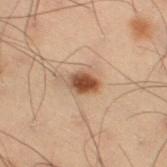Q: Is there a histopathology result?
A: no biopsy performed (imaged during a skin exam)
Q: Where on the body is the lesion?
A: the right thigh
Q: Patient demographics?
A: male, aged 53 to 57
Q: What is the lesion's diameter?
A: ≈3 mm
Q: What is the imaging modality?
A: total-body-photography crop, ~15 mm field of view
Q: How was the tile lit?
A: cross-polarized illumination